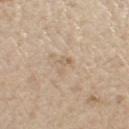This image is a 15 mm lesion crop taken from a total-body photograph.
About 2.5 mm across.
From the left thigh.
Captured under white-light illumination.
The patient is a male aged approximately 70.